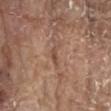workup = total-body-photography surveillance lesion; no biopsy | anatomic site = the chest | tile lighting = white-light illumination | patient = male, about 80 years old | imaging modality = ~15 mm crop, total-body skin-cancer survey.